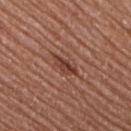Recorded during total-body skin imaging; not selected for excision or biopsy.
The lesion is located on the right upper arm.
Cropped from a whole-body photographic skin survey; the tile spans about 15 mm.
About 3.5 mm across.
The subject is a female aged 73 to 77.